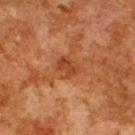Q: How was this image acquired?
A: 15 mm crop, total-body photography
Q: Who is the patient?
A: male, aged 78–82
Q: Lesion location?
A: the upper back
Q: What is the lesion's diameter?
A: about 3.5 mm